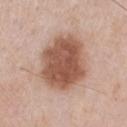biopsy status: no biopsy performed (imaged during a skin exam) | image source: total-body-photography crop, ~15 mm field of view | subject: male, aged 58 to 62 | location: the chest.